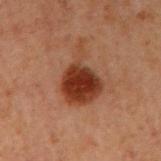Recorded during total-body skin imaging; not selected for excision or biopsy.